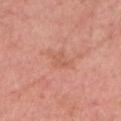No biopsy was performed on this lesion — it was imaged during a full skin examination and was not determined to be concerning.
A female patient roughly 65 years of age.
The recorded lesion diameter is about 3 mm.
An algorithmic analysis of the crop reported a color-variation rating of about 1.5/10 and radial color variation of about 0.5. It also reported a classifier nevus-likeness of about 0/100 and lesion-presence confidence of about 100/100.
On the chest.
This is a white-light tile.
Cropped from a total-body skin-imaging series; the visible field is about 15 mm.